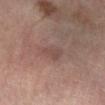workup — catalogued during a skin exam; not biopsied | lesion size — ≈3.5 mm | automated lesion analysis — a footprint of about 6 mm², a shape eccentricity near 0.75, and a shape-asymmetry score of about 0.3 (0 = symmetric); a color-variation rating of about 2/10 and peripheral color asymmetry of about 0.5; an automated nevus-likeness rating near 0 out of 100 and a detector confidence of about 100 out of 100 that the crop contains a lesion | image source — 15 mm crop, total-body photography | patient — male, approximately 70 years of age | location — the right lower leg | lighting — cross-polarized.